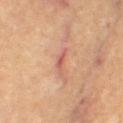Captured during whole-body skin photography for melanoma surveillance; the lesion was not biopsied. About 3.5 mm across. Cropped from a whole-body photographic skin survey; the tile spans about 15 mm. Located on the leg. A female patient, about 65 years old.